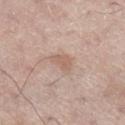acquisition: total-body-photography crop, ~15 mm field of view; subject: male, aged approximately 70; anatomic site: the right lower leg; diameter: ~2.5 mm (longest diameter); lighting: white-light illumination.